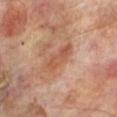Imaged during a routine full-body skin examination; the lesion was not biopsied and no histopathology is available. Automated tile analysis of the lesion measured an area of roughly 7 mm², an eccentricity of roughly 0.9, and a symmetry-axis asymmetry near 0.25. And it measured a border-irregularity rating of about 3/10, internal color variation of about 3 on a 0–10 scale, and peripheral color asymmetry of about 1. The analysis additionally found a nevus-likeness score of about 0/100 and a detector confidence of about 100 out of 100 that the crop contains a lesion. On the leg. A 15 mm close-up extracted from a 3D total-body photography capture. The subject is a male about 70 years old. Approximately 4 mm at its widest.